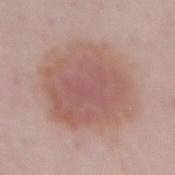– notes · total-body-photography surveillance lesion; no biopsy
– image · 15 mm crop, total-body photography
– automated lesion analysis · a mean CIELAB color near L≈57 a*≈21 b*≈24, about 9 CIELAB-L* units darker than the surrounding skin, and a normalized border contrast of about 6.5; border irregularity of about 2 on a 0–10 scale, a within-lesion color-variation index near 4/10, and radial color variation of about 1
– diameter · ≈8.5 mm
– body site · the mid back
– subject · female, aged around 50